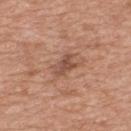notes — total-body-photography surveillance lesion; no biopsy
patient — male, roughly 50 years of age
acquisition — total-body-photography crop, ~15 mm field of view
anatomic site — the upper back
lighting — white-light illumination